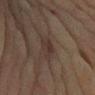Imaged during a routine full-body skin examination; the lesion was not biopsied and no histopathology is available. A female patient about 80 years old. This is a cross-polarized tile. Longest diameter approximately 3 mm. Located on the left upper arm. Cropped from a total-body skin-imaging series; the visible field is about 15 mm.